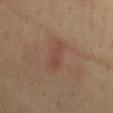notes=total-body-photography surveillance lesion; no biopsy
tile lighting=cross-polarized illumination
automated lesion analysis=a footprint of about 7.5 mm², an eccentricity of roughly 0.9, and a symmetry-axis asymmetry near 0.25; border irregularity of about 3 on a 0–10 scale, internal color variation of about 2.5 on a 0–10 scale, and a peripheral color-asymmetry measure near 1; an automated nevus-likeness rating near 0 out of 100 and a lesion-detection confidence of about 100/100
site=the mid back
image source=~15 mm crop, total-body skin-cancer survey
subject=female, aged around 40